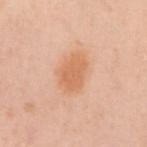biopsy status: imaged on a skin check; not biopsied
tile lighting: white-light illumination
anatomic site: the left upper arm
lesion size: ≈4 mm
TBP lesion metrics: about 9 CIELAB-L* units darker than the surrounding skin and a normalized border contrast of about 6.5; border irregularity of about 2 on a 0–10 scale and a color-variation rating of about 2/10
subject: female, aged approximately 20
image source: ~15 mm tile from a whole-body skin photo